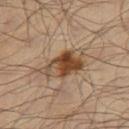This lesion was catalogued during total-body skin photography and was not selected for biopsy.
This is a cross-polarized tile.
A 15 mm crop from a total-body photograph taken for skin-cancer surveillance.
Located on the right thigh.
Measured at roughly 6 mm in maximum diameter.
A male subject roughly 65 years of age.